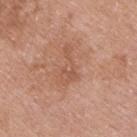{
  "biopsy_status": "not biopsied; imaged during a skin examination"
}